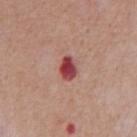biopsy_status: not biopsied; imaged during a skin examination
site: abdomen
image:
  source: total-body photography crop
  field_of_view_mm: 15
patient:
  sex: male
  age_approx: 65